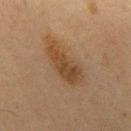Part of a total-body skin-imaging series; this lesion was reviewed on a skin check and was not flagged for biopsy. The tile uses cross-polarized illumination. A 15 mm close-up tile from a total-body photography series done for melanoma screening. Longest diameter approximately 7 mm. The patient is a male about 60 years old. The lesion is on the mid back.